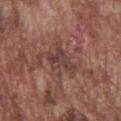Clinical impression:
Recorded during total-body skin imaging; not selected for excision or biopsy.
Image and clinical context:
The recorded lesion diameter is about 4 mm. A roughly 15 mm field-of-view crop from a total-body skin photograph. The lesion is on the chest. The subject is a male aged around 75. The tile uses white-light illumination.